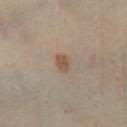Assessment: Imaged during a routine full-body skin examination; the lesion was not biopsied and no histopathology is available. Clinical summary: Captured under cross-polarized illumination. About 2 mm across. A region of skin cropped from a whole-body photographic capture, roughly 15 mm wide. The patient is a male about 40 years old. From the right lower leg.